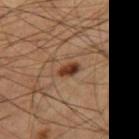Impression: This lesion was catalogued during total-body skin photography and was not selected for biopsy.